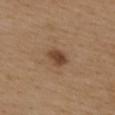The lesion was tiled from a total-body skin photograph and was not biopsied. A female patient, approximately 40 years of age. Captured under white-light illumination. The lesion is on the upper back. A region of skin cropped from a whole-body photographic capture, roughly 15 mm wide. Automated tile analysis of the lesion measured a footprint of about 4.5 mm², an eccentricity of roughly 0.7, and a symmetry-axis asymmetry near 0.2. The analysis additionally found a normalized lesion–skin contrast near 9. Measured at roughly 3 mm in maximum diameter.